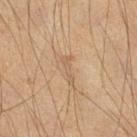Impression:
Part of a total-body skin-imaging series; this lesion was reviewed on a skin check and was not flagged for biopsy.
Acquisition and patient details:
A male subject in their mid- to late 40s. This is a cross-polarized tile. The total-body-photography lesion software estimated a lesion area of about 3 mm² and a shape eccentricity near 0.95. The software also gave a border-irregularity rating of about 7/10. Longest diameter approximately 3 mm. The lesion is on the right lower leg. A close-up tile cropped from a whole-body skin photograph, about 15 mm across.